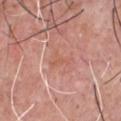biopsy status = catalogued during a skin exam; not biopsied | subject = male, approximately 55 years of age | automated lesion analysis = a lesion area of about 3 mm² | anatomic site = the front of the torso | imaging modality = total-body-photography crop, ~15 mm field of view.